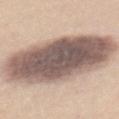• lesion diameter · ≈11.5 mm
• lighting · white-light
• image source · 15 mm crop, total-body photography
• patient · female, aged around 45
• anatomic site · the back